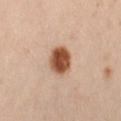biopsy status: imaged on a skin check; not biopsied | automated metrics: an eccentricity of roughly 0.55 and a symmetry-axis asymmetry near 0.1; a lesion color around L≈51 a*≈23 b*≈34 in CIELAB, roughly 18 lightness units darker than nearby skin, and a normalized border contrast of about 13; a border-irregularity rating of about 1/10, a color-variation rating of about 5/10, and radial color variation of about 1.5 | location: the left thigh | diameter: ~3.5 mm (longest diameter) | patient: female, about 40 years old | tile lighting: cross-polarized | acquisition: ~15 mm crop, total-body skin-cancer survey.